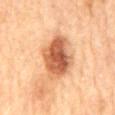{"biopsy_status": "not biopsied; imaged during a skin examination", "patient": {"sex": "male", "age_approx": "85+"}, "lesion_size": {"long_diameter_mm_approx": 6.5}, "site": "back", "automated_metrics": {"area_mm2_approx": 18.0, "eccentricity": 0.85, "shape_asymmetry": 0.2, "cielab_L": 59, "cielab_a": 26, "cielab_b": 38, "vs_skin_darker_L": 18.0, "vs_skin_contrast_norm": 10.5, "border_irregularity_0_10": 2.5, "color_variation_0_10": 7.0, "peripheral_color_asymmetry": 2.0, "nevus_likeness_0_100": 95, "lesion_detection_confidence_0_100": 100}, "image": {"source": "total-body photography crop", "field_of_view_mm": 15}}